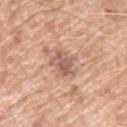Part of a total-body skin-imaging series; this lesion was reviewed on a skin check and was not flagged for biopsy.
The lesion-visualizer software estimated a within-lesion color-variation index near 4.5/10 and a peripheral color-asymmetry measure near 2. And it measured a nevus-likeness score of about 0/100.
The patient is a male roughly 60 years of age.
A 15 mm crop from a total-body photograph taken for skin-cancer surveillance.
Located on the left upper arm.